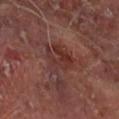<tbp_lesion>
<biopsy_status>not biopsied; imaged during a skin examination</biopsy_status>
</tbp_lesion>